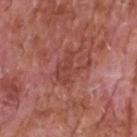{
  "biopsy_status": "not biopsied; imaged during a skin examination",
  "site": "head or neck",
  "image": {
    "source": "total-body photography crop",
    "field_of_view_mm": 15
  },
  "lesion_size": {
    "long_diameter_mm_approx": 4.0
  },
  "automated_metrics": {
    "shape_asymmetry": 0.55,
    "vs_skin_darker_L": 7.0,
    "nevus_likeness_0_100": 0,
    "lesion_detection_confidence_0_100": 100
  },
  "patient": {
    "sex": "male",
    "age_approx": 60
  }
}